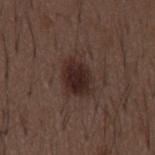The lesion was photographed on a routine skin check and not biopsied; there is no pathology result. A male subject roughly 50 years of age. This is a white-light tile. About 5 mm across. A 15 mm close-up extracted from a 3D total-body photography capture. Located on the mid back.